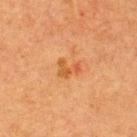{"biopsy_status": "not biopsied; imaged during a skin examination", "lesion_size": {"long_diameter_mm_approx": 2.5}, "site": "upper back", "image": {"source": "total-body photography crop", "field_of_view_mm": 15}, "patient": {"sex": "male", "age_approx": 40}, "lighting": "cross-polarized"}